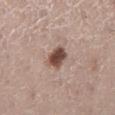{"biopsy_status": "not biopsied; imaged during a skin examination", "lesion_size": {"long_diameter_mm_approx": 3.0}, "site": "right lower leg", "image": {"source": "total-body photography crop", "field_of_view_mm": 15}, "lighting": "white-light", "automated_metrics": {"shape_asymmetry": 0.25, "nevus_likeness_0_100": 95, "lesion_detection_confidence_0_100": 100}, "patient": {"sex": "female", "age_approx": 45}}